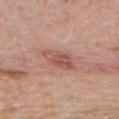<case>
<biopsy_status>not biopsied; imaged during a skin examination</biopsy_status>
<automated_metrics>
  <eccentricity>0.85</eccentricity>
  <shape_asymmetry>0.25</shape_asymmetry>
  <nevus_likeness_0_100>10</nevus_likeness_0_100>
  <lesion_detection_confidence_0_100>100</lesion_detection_confidence_0_100>
</automated_metrics>
<image>
  <source>total-body photography crop</source>
  <field_of_view_mm>15</field_of_view_mm>
</image>
<lesion_size>
  <long_diameter_mm_approx>4.0</long_diameter_mm_approx>
</lesion_size>
<patient>
  <sex>male</sex>
  <age_approx>40</age_approx>
</patient>
<lighting>white-light</lighting>
<site>upper back</site>
</case>